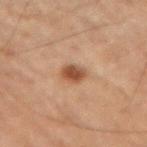No biopsy was performed on this lesion — it was imaged during a full skin examination and was not determined to be concerning. About 2.5 mm across. The lesion is located on the right upper arm. The tile uses cross-polarized illumination. Cropped from a total-body skin-imaging series; the visible field is about 15 mm. A male patient, aged around 70.